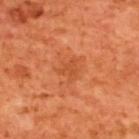Findings:
* lesion size · about 2.5 mm
* lighting · cross-polarized illumination
* anatomic site · the upper back
* image source · ~15 mm tile from a whole-body skin photo
* subject · male, in their mid-60s
* image-analysis metrics · a footprint of about 4.5 mm² and a shape-asymmetry score of about 0.45 (0 = symmetric); a border-irregularity rating of about 4.5/10 and peripheral color asymmetry of about 0.5; a nevus-likeness score of about 20/100 and a detector confidence of about 100 out of 100 that the crop contains a lesion